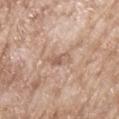  biopsy_status: not biopsied; imaged during a skin examination